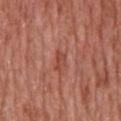• notes · catalogued during a skin exam; not biopsied
• anatomic site · the chest
• tile lighting · white-light
• acquisition · ~15 mm crop, total-body skin-cancer survey
• subject · male, about 65 years old